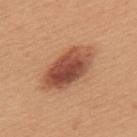Q: What did automated image analysis measure?
A: a footprint of about 19 mm², an eccentricity of roughly 0.85, and a symmetry-axis asymmetry near 0.15
Q: How was this image acquired?
A: ~15 mm tile from a whole-body skin photo
Q: Lesion location?
A: the upper back
Q: What lighting was used for the tile?
A: white-light illumination
Q: Patient demographics?
A: female, in their 30s
Q: What is the lesion's diameter?
A: about 7 mm